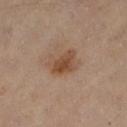Captured during whole-body skin photography for melanoma surveillance; the lesion was not biopsied. A 15 mm close-up tile from a total-body photography series done for melanoma screening. A female subject roughly 60 years of age. Located on the leg.